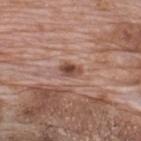No biopsy was performed on this lesion — it was imaged during a full skin examination and was not determined to be concerning.